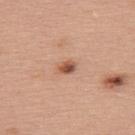imaging modality — ~15 mm crop, total-body skin-cancer survey
body site — the back
lighting — white-light
subject — female, roughly 60 years of age
diameter — ≈2.5 mm
automated lesion analysis — roughly 13 lightness units darker than nearby skin and a normalized border contrast of about 8.5; an automated nevus-likeness rating near 95 out of 100 and a lesion-detection confidence of about 100/100